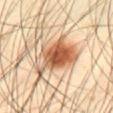| feature | finding |
|---|---|
| notes | total-body-photography surveillance lesion; no biopsy |
| image-analysis metrics | an average lesion color of about L≈61 a*≈21 b*≈36 (CIELAB), a lesion–skin lightness drop of about 17, and a normalized lesion–skin contrast near 10.5; peripheral color asymmetry of about 3 |
| acquisition | 15 mm crop, total-body photography |
| lesion size | about 6 mm |
| patient | male, roughly 40 years of age |
| lighting | cross-polarized illumination |
| location | the abdomen |